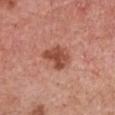Captured during whole-body skin photography for melanoma surveillance; the lesion was not biopsied. Captured under white-light illumination. Located on the chest. The recorded lesion diameter is about 3.5 mm. A female patient aged 58 to 62. A 15 mm close-up tile from a total-body photography series done for melanoma screening.